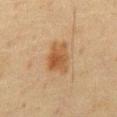biopsy status: total-body-photography surveillance lesion; no biopsy | body site: the abdomen | lighting: cross-polarized | image-analysis metrics: a lesion color around L≈41 a*≈17 b*≈31 in CIELAB, about 8 CIELAB-L* units darker than the surrounding skin, and a normalized lesion–skin contrast near 8; a color-variation rating of about 4/10 and a peripheral color-asymmetry measure near 1.5 | subject: male, aged 58 to 62 | imaging modality: ~15 mm tile from a whole-body skin photo.